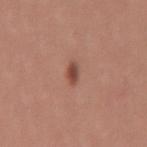The subject is a male about 30 years old. An algorithmic analysis of the crop reported border irregularity of about 2.5 on a 0–10 scale and a within-lesion color-variation index near 1.5/10. And it measured a classifier nevus-likeness of about 100/100 and a lesion-detection confidence of about 100/100. Approximately 2.5 mm at its widest. Captured under white-light illumination. The lesion is located on the mid back. A 15 mm crop from a total-body photograph taken for skin-cancer surveillance.